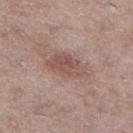Context:
A female subject roughly 60 years of age. The recorded lesion diameter is about 5.5 mm. Captured under white-light illumination. This image is a 15 mm lesion crop taken from a total-body photograph. The lesion is on the leg.